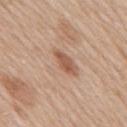site: mid back
lighting: white-light
image:
  source: total-body photography crop
  field_of_view_mm: 15
lesion_size:
  long_diameter_mm_approx: 4.0
patient:
  sex: male
  age_approx: 60